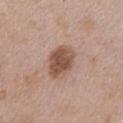Part of a total-body skin-imaging series; this lesion was reviewed on a skin check and was not flagged for biopsy.
Approximately 4.5 mm at its widest.
Captured under white-light illumination.
The subject is a female in their mid-70s.
An algorithmic analysis of the crop reported a mean CIELAB color near L≈51 a*≈20 b*≈28. The analysis additionally found border irregularity of about 2 on a 0–10 scale and peripheral color asymmetry of about 1. It also reported an automated nevus-likeness rating near 55 out of 100 and lesion-presence confidence of about 100/100.
Located on the front of the torso.
A close-up tile cropped from a whole-body skin photograph, about 15 mm across.